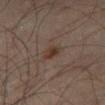* notes: no biopsy performed (imaged during a skin exam)
* tile lighting: cross-polarized illumination
* patient: male, about 65 years old
* automated lesion analysis: an area of roughly 3 mm², a shape eccentricity near 0.75, and a shape-asymmetry score of about 0.2 (0 = symmetric); an automated nevus-likeness rating near 75 out of 100 and a detector confidence of about 100 out of 100 that the crop contains a lesion
* lesion diameter: ≈2 mm
* anatomic site: the leg
* image source: 15 mm crop, total-body photography